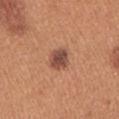{
  "biopsy_status": "not biopsied; imaged during a skin examination",
  "lighting": "white-light",
  "site": "right upper arm",
  "patient": {
    "sex": "female",
    "age_approx": 40
  },
  "lesion_size": {
    "long_diameter_mm_approx": 3.0
  },
  "image": {
    "source": "total-body photography crop",
    "field_of_view_mm": 15
  }
}